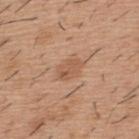Q: Was a biopsy performed?
A: no biopsy performed (imaged during a skin exam)
Q: What is the anatomic site?
A: the upper back
Q: How was this image acquired?
A: 15 mm crop, total-body photography
Q: What is the lesion's diameter?
A: about 3.5 mm
Q: Illumination type?
A: white-light
Q: Patient demographics?
A: male, aged 38–42